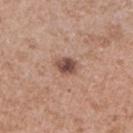A female subject about 30 years old. Located on the right upper arm. The tile uses white-light illumination. A region of skin cropped from a whole-body photographic capture, roughly 15 mm wide. Longest diameter approximately 2.5 mm.